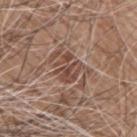<tbp_lesion>
  <biopsy_status>not biopsied; imaged during a skin examination</biopsy_status>
  <patient>
    <sex>male</sex>
    <age_approx>60</age_approx>
  </patient>
  <image>
    <source>total-body photography crop</source>
    <field_of_view_mm>15</field_of_view_mm>
  </image>
  <site>right upper arm</site>
</tbp_lesion>